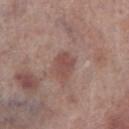Notes:
• biopsy status · no biopsy performed (imaged during a skin exam)
• diameter · about 3 mm
• image · total-body-photography crop, ~15 mm field of view
• automated lesion analysis · an average lesion color of about L≈48 a*≈21 b*≈23 (CIELAB), roughly 9 lightness units darker than nearby skin, and a normalized lesion–skin contrast near 6.5; a color-variation rating of about 2.5/10 and radial color variation of about 1
• patient · male, approximately 70 years of age
• anatomic site · the right lower leg
• tile lighting · white-light